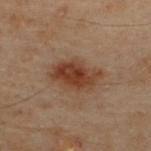| field | value |
|---|---|
| workup | imaged on a skin check; not biopsied |
| patient | male, aged 48–52 |
| illumination | cross-polarized illumination |
| acquisition | total-body-photography crop, ~15 mm field of view |
| body site | the upper back |